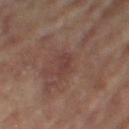Clinical impression:
Captured during whole-body skin photography for melanoma surveillance; the lesion was not biopsied.
Clinical summary:
Automated tile analysis of the lesion measured a lesion color around L≈37 a*≈19 b*≈21 in CIELAB, roughly 6 lightness units darker than nearby skin, and a lesion-to-skin contrast of about 5 (normalized; higher = more distinct). Longest diameter approximately 2.5 mm. A close-up tile cropped from a whole-body skin photograph, about 15 mm across. A female patient aged 63–67. Imaged with cross-polarized lighting. Located on the left leg.